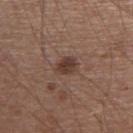Assessment:
The lesion was photographed on a routine skin check and not biopsied; there is no pathology result.
Acquisition and patient details:
A 15 mm crop from a total-body photograph taken for skin-cancer surveillance. A male subject, aged 53–57. The lesion's longest dimension is about 3 mm. Captured under white-light illumination. The lesion is on the right upper arm.